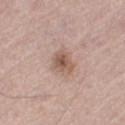Assessment:
Captured during whole-body skin photography for melanoma surveillance; the lesion was not biopsied.
Context:
A roughly 15 mm field-of-view crop from a total-body skin photograph. A male subject, in their mid- to late 50s. This is a white-light tile. The total-body-photography lesion software estimated a footprint of about 5.5 mm², an outline eccentricity of about 0.5 (0 = round, 1 = elongated), and a symmetry-axis asymmetry near 0.35. The software also gave a lesion color around L≈56 a*≈18 b*≈26 in CIELAB, roughly 10 lightness units darker than nearby skin, and a lesion-to-skin contrast of about 7.5 (normalized; higher = more distinct). And it measured a nevus-likeness score of about 50/100. From the right thigh.